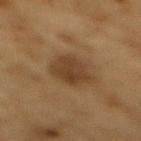Captured during whole-body skin photography for melanoma surveillance; the lesion was not biopsied. From the mid back. Longest diameter approximately 4 mm. This is a cross-polarized tile. The patient is a male in their mid-80s or older. A roughly 15 mm field-of-view crop from a total-body skin photograph.